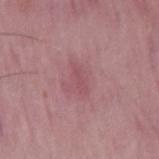Assessment:
Imaged during a routine full-body skin examination; the lesion was not biopsied and no histopathology is available.
Context:
A close-up tile cropped from a whole-body skin photograph, about 15 mm across. The lesion-visualizer software estimated an area of roughly 3 mm² and a shape-asymmetry score of about 0.25 (0 = symmetric). It also reported about 6 CIELAB-L* units darker than the surrounding skin and a lesion-to-skin contrast of about 4.5 (normalized; higher = more distinct). And it measured border irregularity of about 3.5 on a 0–10 scale and a peripheral color-asymmetry measure near 0. It also reported an automated nevus-likeness rating near 0 out of 100 and lesion-presence confidence of about 90/100. This is a white-light tile. The recorded lesion diameter is about 3 mm. From the mid back. The patient is a male aged around 50.